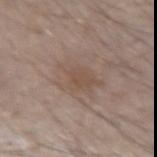workup: imaged on a skin check; not biopsied
imaging modality: ~15 mm crop, total-body skin-cancer survey
subject: male, aged around 70
lesion diameter: about 5 mm
illumination: white-light
anatomic site: the left forearm